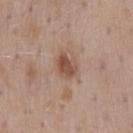Imaged during a routine full-body skin examination; the lesion was not biopsied and no histopathology is available.
From the mid back.
A lesion tile, about 15 mm wide, cut from a 3D total-body photograph.
The subject is a male roughly 55 years of age.
The tile uses white-light illumination.
Automated image analysis of the tile measured a shape eccentricity near 0.75 and two-axis asymmetry of about 0.3. And it measured a mean CIELAB color near L≈50 a*≈20 b*≈28 and roughly 11 lightness units darker than nearby skin. It also reported border irregularity of about 2.5 on a 0–10 scale, a within-lesion color-variation index near 3.5/10, and peripheral color asymmetry of about 1. The software also gave an automated nevus-likeness rating near 85 out of 100 and a lesion-detection confidence of about 100/100.
Approximately 3 mm at its widest.